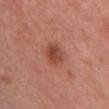<tbp_lesion>
<biopsy_status>not biopsied; imaged during a skin examination</biopsy_status>
<image>
  <source>total-body photography crop</source>
  <field_of_view_mm>15</field_of_view_mm>
</image>
<site>chest</site>
<patient>
  <sex>female</sex>
  <age_approx>35</age_approx>
</patient>
</tbp_lesion>